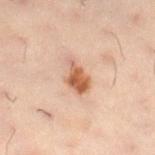Case summary:
- follow-up · catalogued during a skin exam; not biopsied
- illumination · cross-polarized
- automated lesion analysis · a lesion area of about 7 mm², an eccentricity of roughly 0.8, and a shape-asymmetry score of about 0.3 (0 = symmetric); a border-irregularity index near 3/10 and a within-lesion color-variation index near 5/10; an automated nevus-likeness rating near 90 out of 100
- lesion size · ≈4 mm
- image source · ~15 mm crop, total-body skin-cancer survey
- subject · female, approximately 30 years of age
- anatomic site · the leg